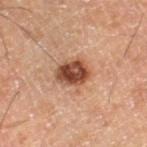The subject is a male aged approximately 60.
This image is a 15 mm lesion crop taken from a total-body photograph.
On the left thigh.